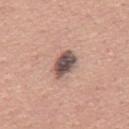notes=no biopsy performed (imaged during a skin exam) | patient=male, approximately 45 years of age | imaging modality=~15 mm crop, total-body skin-cancer survey | site=the back | diameter=≈4 mm.